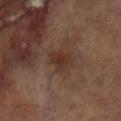follow-up: catalogued during a skin exam; not biopsied | illumination: cross-polarized illumination | body site: the right arm | subject: female, in their 80s | imaging modality: 15 mm crop, total-body photography.